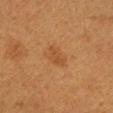Q: Was a biopsy performed?
A: total-body-photography surveillance lesion; no biopsy
Q: How was this image acquired?
A: 15 mm crop, total-body photography
Q: What lighting was used for the tile?
A: cross-polarized illumination
Q: Where on the body is the lesion?
A: the right forearm
Q: Automated lesion metrics?
A: a footprint of about 5.5 mm² and a shape-asymmetry score of about 0.3 (0 = symmetric)
Q: What are the patient's age and sex?
A: female, approximately 40 years of age